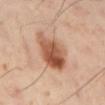automated_metrics:
  nevus_likeness_0_100: 100
  lesion_detection_confidence_0_100: 100
image:
  source: total-body photography crop
  field_of_view_mm: 15
lighting: cross-polarized
patient:
  sex: male
  age_approx: 40
site: left lower leg